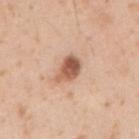notes: imaged on a skin check; not biopsied | image source: 15 mm crop, total-body photography | subject: male, aged approximately 30 | location: the right upper arm | lighting: white-light.